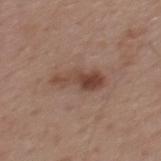biopsy_status: not biopsied; imaged during a skin examination
image:
  source: total-body photography crop
  field_of_view_mm: 15
lighting: white-light
automated_metrics:
  area_mm2_approx: 8.5
  eccentricity: 0.95
  shape_asymmetry: 0.35
  cielab_L: 45
  cielab_a: 19
  cielab_b: 26
  vs_skin_darker_L: 10.0
  vs_skin_contrast_norm: 8.0
lesion_size:
  long_diameter_mm_approx: 5.5
patient:
  sex: male
  age_approx: 55
site: mid back